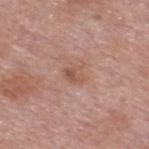The lesion was tiled from a total-body skin photograph and was not biopsied. On the upper back. A roughly 15 mm field-of-view crop from a total-body skin photograph. The total-body-photography lesion software estimated border irregularity of about 3.5 on a 0–10 scale, internal color variation of about 4.5 on a 0–10 scale, and radial color variation of about 1.5. And it measured a classifier nevus-likeness of about 5/100 and lesion-presence confidence of about 100/100. Measured at roughly 2.5 mm in maximum diameter. A male subject, in their mid- to late 50s.